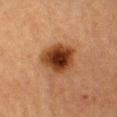Findings:
• follow-up — catalogued during a skin exam; not biopsied
• illumination — cross-polarized illumination
• acquisition — total-body-photography crop, ~15 mm field of view
• automated metrics — a border-irregularity index near 2/10 and a peripheral color-asymmetry measure near 1.5; a classifier nevus-likeness of about 100/100 and a lesion-detection confidence of about 100/100
• site — the chest
• size — ≈4.5 mm
• subject — female, approximately 40 years of age